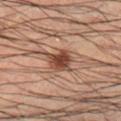<lesion>
  <biopsy_status>not biopsied; imaged during a skin examination</biopsy_status>
  <lighting>cross-polarized</lighting>
  <image>
    <source>total-body photography crop</source>
    <field_of_view_mm>15</field_of_view_mm>
  </image>
  <lesion_size>
    <long_diameter_mm_approx>4.0</long_diameter_mm_approx>
  </lesion_size>
  <site>right lower leg</site>
  <patient>
    <sex>male</sex>
    <age_approx>50</age_approx>
  </patient>
  <automated_metrics>
    <cielab_L>33</cielab_L>
    <cielab_a>17</cielab_a>
    <cielab_b>22</cielab_b>
    <vs_skin_darker_L>11.0</vs_skin_darker_L>
    <vs_skin_contrast_norm>10.0</vs_skin_contrast_norm>
    <border_irregularity_0_10>3.0</border_irregularity_0_10>
    <color_variation_0_10>3.0</color_variation_0_10>
    <peripheral_color_asymmetry>1.0</peripheral_color_asymmetry>
  </automated_metrics>
</lesion>